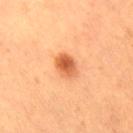The lesion was photographed on a routine skin check and not biopsied; there is no pathology result.
From the left leg.
Cropped from a total-body skin-imaging series; the visible field is about 15 mm.
The patient is a female roughly 45 years of age.